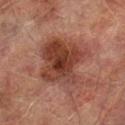A 15 mm close-up extracted from a 3D total-body photography capture. On the right lower leg. Automated image analysis of the tile measured an average lesion color of about L≈33 a*≈21 b*≈24 (CIELAB), roughly 10 lightness units darker than nearby skin, and a lesion-to-skin contrast of about 9 (normalized; higher = more distinct). The analysis additionally found a color-variation rating of about 5.5/10 and radial color variation of about 2. The analysis additionally found a lesion-detection confidence of about 100/100. This is a cross-polarized tile. Measured at roughly 6.5 mm in maximum diameter. A male patient, aged around 75.